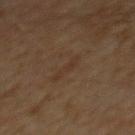Q: Was this lesion biopsied?
A: total-body-photography surveillance lesion; no biopsy
Q: What kind of image is this?
A: ~15 mm crop, total-body skin-cancer survey
Q: Lesion location?
A: the mid back
Q: Who is the patient?
A: male, roughly 60 years of age